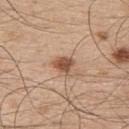notes: imaged on a skin check; not biopsied | anatomic site: the back | diameter: ≈2.5 mm | subject: male, approximately 50 years of age | lighting: white-light illumination | acquisition: total-body-photography crop, ~15 mm field of view | automated lesion analysis: a footprint of about 4.5 mm² and an eccentricity of roughly 0.55; an average lesion color of about L≈52 a*≈20 b*≈31 (CIELAB), about 14 CIELAB-L* units darker than the surrounding skin, and a normalized lesion–skin contrast near 9.5; internal color variation of about 3 on a 0–10 scale and radial color variation of about 1; a detector confidence of about 100 out of 100 that the crop contains a lesion.